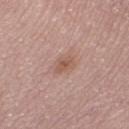Part of a total-body skin-imaging series; this lesion was reviewed on a skin check and was not flagged for biopsy. Longest diameter approximately 2.5 mm. The tile uses white-light illumination. Located on the right lower leg. A 15 mm close-up tile from a total-body photography series done for melanoma screening. The subject is a male aged 58–62.